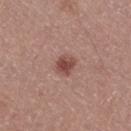Captured during whole-body skin photography for melanoma surveillance; the lesion was not biopsied. Located on the right thigh. The subject is a male in their mid- to late 60s. A close-up tile cropped from a whole-body skin photograph, about 15 mm across.